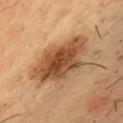{"patient": {"sex": "male", "age_approx": 35}, "lesion_size": {"long_diameter_mm_approx": 8.5}, "automated_metrics": {"area_mm2_approx": 26.0, "cielab_L": 45, "cielab_a": 20, "cielab_b": 33, "vs_skin_contrast_norm": 10.0}, "site": "chest", "lighting": "cross-polarized", "image": {"source": "total-body photography crop", "field_of_view_mm": 15}}